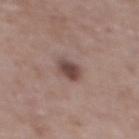Case summary:
- follow-up: total-body-photography surveillance lesion; no biopsy
- patient: female, roughly 65 years of age
- body site: the upper back
- lesion diameter: ≈3 mm
- image: 15 mm crop, total-body photography
- tile lighting: white-light